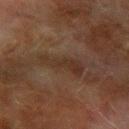{"biopsy_status": "not biopsied; imaged during a skin examination", "lighting": "cross-polarized", "automated_metrics": {"border_irregularity_0_10": 5.0, "peripheral_color_asymmetry": 1.0}, "site": "left forearm", "patient": {"sex": "male", "age_approx": 70}, "image": {"source": "total-body photography crop", "field_of_view_mm": 15}, "lesion_size": {"long_diameter_mm_approx": 4.5}}